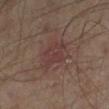The lesion was photographed on a routine skin check and not biopsied; there is no pathology result. A patient aged 53–57. A 15 mm close-up tile from a total-body photography series done for melanoma screening. Captured under cross-polarized illumination. Measured at roughly 4 mm in maximum diameter. The lesion is on the left lower leg.